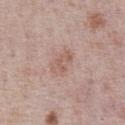A male subject aged approximately 75. From the chest. This is a white-light tile. The lesion-visualizer software estimated an area of roughly 5.5 mm² and a symmetry-axis asymmetry near 0.25. A 15 mm close-up tile from a total-body photography series done for melanoma screening. The recorded lesion diameter is about 3.5 mm.